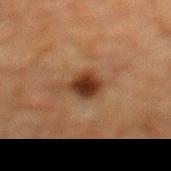follow-up: catalogued during a skin exam; not biopsied
body site: the mid back
subject: male, aged 58–62
lighting: cross-polarized
diameter: ≈2.5 mm
image: 15 mm crop, total-body photography